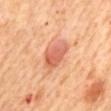Background: Located on the mid back. Automated image analysis of the tile measured a lesion color around L≈61 a*≈29 b*≈35 in CIELAB and a lesion-to-skin contrast of about 7 (normalized; higher = more distinct). The software also gave border irregularity of about 2.5 on a 0–10 scale, a color-variation rating of about 4/10, and a peripheral color-asymmetry measure near 1. A 15 mm crop from a total-body photograph taken for skin-cancer surveillance. The tile uses cross-polarized illumination. The patient is a female aged 63–67. Measured at roughly 4 mm in maximum diameter.